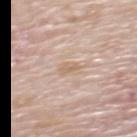• automated lesion analysis · an eccentricity of roughly 0.85 and a shape-asymmetry score of about 0.4 (0 = symmetric); a lesion–skin lightness drop of about 7 and a normalized lesion–skin contrast near 5.5; an automated nevus-likeness rating near 0 out of 100
• image · 15 mm crop, total-body photography
• anatomic site · the upper back
• illumination · white-light
• subject · female, aged 63 to 67